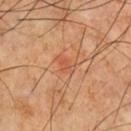• workup — imaged on a skin check; not biopsied
• patient — male, aged 63 to 67
• automated lesion analysis — a footprint of about 3 mm², a shape eccentricity near 0.85, and a shape-asymmetry score of about 0.45 (0 = symmetric); a lesion color around L≈52 a*≈27 b*≈35 in CIELAB, a lesion–skin lightness drop of about 6, and a normalized lesion–skin contrast near 5
• body site — the front of the torso
• illumination — cross-polarized illumination
• imaging modality — total-body-photography crop, ~15 mm field of view
• diameter — about 2.5 mm